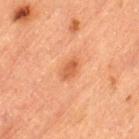workup: imaged on a skin check; not biopsied | tile lighting: cross-polarized | image-analysis metrics: a lesion area of about 4 mm² and an eccentricity of roughly 0.75; a classifier nevus-likeness of about 40/100 and lesion-presence confidence of about 100/100 | patient: male, aged around 85 or older | lesion diameter: ≈3 mm | location: the left thigh | imaging modality: ~15 mm tile from a whole-body skin photo.